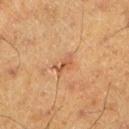| key | value |
|---|---|
| workup | catalogued during a skin exam; not biopsied |
| image source | total-body-photography crop, ~15 mm field of view |
| patient | male, roughly 60 years of age |
| size | ~2.5 mm (longest diameter) |
| TBP lesion metrics | a lesion area of about 3 mm² and a symmetry-axis asymmetry near 0.45 |
| body site | the left lower leg |